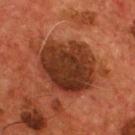notes: imaged on a skin check; not biopsied
anatomic site: the chest
tile lighting: cross-polarized illumination
lesion diameter: ~9.5 mm (longest diameter)
image source: 15 mm crop, total-body photography
TBP lesion metrics: a shape eccentricity near 0.65; border irregularity of about 4 on a 0–10 scale, internal color variation of about 5 on a 0–10 scale, and a peripheral color-asymmetry measure near 1.5; an automated nevus-likeness rating near 65 out of 100 and a lesion-detection confidence of about 100/100
patient: male, approximately 55 years of age